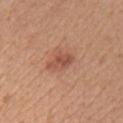{
  "biopsy_status": "not biopsied; imaged during a skin examination",
  "patient": {
    "sex": "female",
    "age_approx": 45
  },
  "lighting": "white-light",
  "image": {
    "source": "total-body photography crop",
    "field_of_view_mm": 15
  },
  "site": "chest"
}